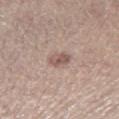| feature | finding |
|---|---|
| follow-up | catalogued during a skin exam; not biopsied |
| diameter | ≈2.5 mm |
| subject | male, in their mid-60s |
| anatomic site | the right thigh |
| image-analysis metrics | an area of roughly 4 mm² and a symmetry-axis asymmetry near 0.25; border irregularity of about 2 on a 0–10 scale, a color-variation rating of about 3.5/10, and a peripheral color-asymmetry measure near 1.5; lesion-presence confidence of about 100/100 |
| illumination | white-light |
| image source | ~15 mm crop, total-body skin-cancer survey |